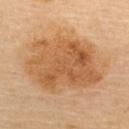Impression: This lesion was catalogued during total-body skin photography and was not selected for biopsy. Clinical summary: A close-up tile cropped from a whole-body skin photograph, about 15 mm across. The total-body-photography lesion software estimated an outline eccentricity of about 0.75 (0 = round, 1 = elongated) and two-axis asymmetry of about 0.2. And it measured a border-irregularity rating of about 2.5/10, internal color variation of about 6 on a 0–10 scale, and a peripheral color-asymmetry measure near 2. The lesion is located on the upper back. Imaged with cross-polarized lighting. The subject is a female in their 60s.